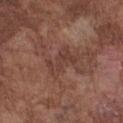The lesion was photographed on a routine skin check and not biopsied; there is no pathology result.
On the chest.
A male patient, aged 73 to 77.
A roughly 15 mm field-of-view crop from a total-body skin photograph.
Approximately 4 mm at its widest.
The total-body-photography lesion software estimated a lesion area of about 7 mm² and an eccentricity of roughly 0.85. And it measured a border-irregularity index near 6/10, internal color variation of about 1.5 on a 0–10 scale, and radial color variation of about 0.5. The software also gave an automated nevus-likeness rating near 0 out of 100.
Captured under white-light illumination.